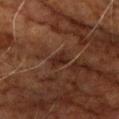Case summary:
– follow-up · catalogued during a skin exam; not biopsied
– body site · the right upper arm
– image source · 15 mm crop, total-body photography
– patient · male, approximately 70 years of age
– automated metrics · a lesion–skin lightness drop of about 6; border irregularity of about 2 on a 0–10 scale and a peripheral color-asymmetry measure near 0.5